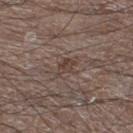- workup — no biopsy performed (imaged during a skin exam)
- illumination — white-light
- patient — male, aged 63 to 67
- image source — ~15 mm tile from a whole-body skin photo
- diameter — ≈2.5 mm
- body site — the left lower leg